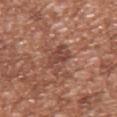{
  "patient": {
    "sex": "male",
    "age_approx": 45
  },
  "image": {
    "source": "total-body photography crop",
    "field_of_view_mm": 15
  },
  "site": "left upper arm",
  "lesion_size": {
    "long_diameter_mm_approx": 4.5
  },
  "lighting": "white-light",
  "automated_metrics": {
    "eccentricity": 0.85,
    "shape_asymmetry": 0.35,
    "border_irregularity_0_10": 5.0,
    "color_variation_0_10": 2.5,
    "peripheral_color_asymmetry": 1.0,
    "nevus_likeness_0_100": 0,
    "lesion_detection_confidence_0_100": 100
  }
}